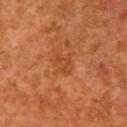The lesion-visualizer software estimated an area of roughly 5 mm², a shape eccentricity near 0.65, and a symmetry-axis asymmetry near 0.25. The software also gave an average lesion color of about L≈38 a*≈24 b*≈34 (CIELAB), about 5 CIELAB-L* units darker than the surrounding skin, and a normalized lesion–skin contrast near 4.5. And it measured a nevus-likeness score of about 0/100.
Captured under cross-polarized illumination.
On the right upper arm.
A lesion tile, about 15 mm wide, cut from a 3D total-body photograph.
A female patient about 50 years old.
About 3 mm across.